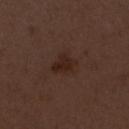The lesion was tiled from a total-body skin photograph and was not biopsied. A female subject aged 48 to 52. A 15 mm crop from a total-body photograph taken for skin-cancer surveillance. From the left upper arm. The lesion-visualizer software estimated border irregularity of about 3.5 on a 0–10 scale, a within-lesion color-variation index near 2.5/10, and peripheral color asymmetry of about 1. And it measured a classifier nevus-likeness of about 80/100 and a lesion-detection confidence of about 100/100.